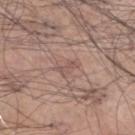This lesion was catalogued during total-body skin photography and was not selected for biopsy.
Automated image analysis of the tile measured a mean CIELAB color near L≈53 a*≈19 b*≈23, roughly 7 lightness units darker than nearby skin, and a normalized border contrast of about 5. The analysis additionally found a border-irregularity rating of about 5/10 and a within-lesion color-variation index near 0/10. The software also gave a nevus-likeness score of about 0/100 and a detector confidence of about 90 out of 100 that the crop contains a lesion.
A close-up tile cropped from a whole-body skin photograph, about 15 mm across.
A male patient, aged around 65.
Located on the head or neck.
Captured under white-light illumination.